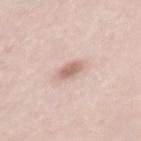acquisition — ~15 mm crop, total-body skin-cancer survey | patient — female, roughly 30 years of age | body site — the back | automated lesion analysis — an area of roughly 4.5 mm², an eccentricity of roughly 0.8, and a shape-asymmetry score of about 0.25 (0 = symmetric); a border-irregularity index near 2.5/10, a color-variation rating of about 2/10, and radial color variation of about 0.5; a classifier nevus-likeness of about 85/100 and lesion-presence confidence of about 100/100.